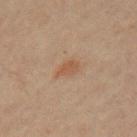{"biopsy_status": "not biopsied; imaged during a skin examination", "image": {"source": "total-body photography crop", "field_of_view_mm": 15}, "patient": {"sex": "female", "age_approx": 45}, "site": "left upper arm"}